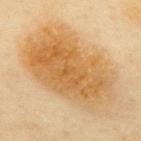Impression:
The lesion was tiled from a total-body skin photograph and was not biopsied.
Image and clinical context:
Automated image analysis of the tile measured a shape eccentricity near 0.8 and two-axis asymmetry of about 0.15. It also reported a mean CIELAB color near L≈58 a*≈17 b*≈40, about 10 CIELAB-L* units darker than the surrounding skin, and a normalized border contrast of about 8. The software also gave a detector confidence of about 100 out of 100 that the crop contains a lesion. On the mid back. A region of skin cropped from a whole-body photographic capture, roughly 15 mm wide. The recorded lesion diameter is about 12 mm. A female subject aged 58–62.